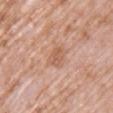<lesion>
  <biopsy_status>not biopsied; imaged during a skin examination</biopsy_status>
  <site>front of the torso</site>
  <patient>
    <sex>female</sex>
    <age_approx>70</age_approx>
  </patient>
  <image>
    <source>total-body photography crop</source>
    <field_of_view_mm>15</field_of_view_mm>
  </image>
</lesion>